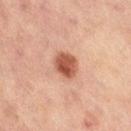Impression: Imaged during a routine full-body skin examination; the lesion was not biopsied and no histopathology is available. Image and clinical context: This is a cross-polarized tile. This image is a 15 mm lesion crop taken from a total-body photograph. A female subject aged 58 to 62. On the right thigh.